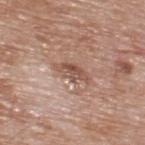  biopsy_status: not biopsied; imaged during a skin examination
  lighting: white-light
  image:
    source: total-body photography crop
    field_of_view_mm: 15
  automated_metrics:
    cielab_L: 52
    cielab_a: 20
    cielab_b: 27
    vs_skin_contrast_norm: 7.0
    color_variation_0_10: 4.5
    peripheral_color_asymmetry: 1.5
    lesion_detection_confidence_0_100: 100
  site: back
  lesion_size:
    long_diameter_mm_approx: 4.0
  patient:
    sex: male
    age_approx: 60